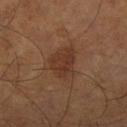The lesion was photographed on a routine skin check and not biopsied; there is no pathology result. A male subject, aged 63 to 67. The lesion is on the leg. This is a cross-polarized tile. Measured at roughly 4.5 mm in maximum diameter. Automated image analysis of the tile measured an area of roughly 11 mm², an eccentricity of roughly 0.7, and two-axis asymmetry of about 0.3. The software also gave a border-irregularity rating of about 3/10, internal color variation of about 2.5 on a 0–10 scale, and peripheral color asymmetry of about 1. It also reported a nevus-likeness score of about 65/100 and a lesion-detection confidence of about 100/100. A lesion tile, about 15 mm wide, cut from a 3D total-body photograph.